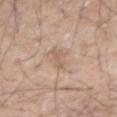Background:
An algorithmic analysis of the crop reported an area of roughly 3 mm² and a shape-asymmetry score of about 0.35 (0 = symmetric). The analysis additionally found an average lesion color of about L≈60 a*≈17 b*≈28 (CIELAB), roughly 8 lightness units darker than nearby skin, and a normalized border contrast of about 5. And it measured an automated nevus-likeness rating near 0 out of 100 and a detector confidence of about 85 out of 100 that the crop contains a lesion. A male patient, aged 68 to 72. The lesion's longest dimension is about 3.5 mm. Located on the right forearm. A lesion tile, about 15 mm wide, cut from a 3D total-body photograph. Imaged with white-light lighting.